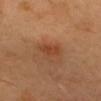Background:
A 15 mm close-up extracted from a 3D total-body photography capture. A female subject aged 38–42. Located on the head or neck. The lesion-visualizer software estimated an area of roughly 4.5 mm², a shape eccentricity near 0.8, and a shape-asymmetry score of about 0.2 (0 = symmetric). The analysis additionally found an average lesion color of about L≈44 a*≈25 b*≈36 (CIELAB) and a lesion–skin lightness drop of about 8. The tile uses cross-polarized illumination. Measured at roughly 3 mm in maximum diameter.